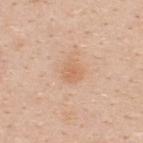Impression:
Imaged during a routine full-body skin examination; the lesion was not biopsied and no histopathology is available.
Background:
Cropped from a whole-body photographic skin survey; the tile spans about 15 mm. Captured under white-light illumination. From the back. The subject is a female aged 28–32.